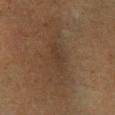Clinical impression:
The lesion was photographed on a routine skin check and not biopsied; there is no pathology result.
Background:
On the right lower leg. A close-up tile cropped from a whole-body skin photograph, about 15 mm across. A male patient roughly 60 years of age.